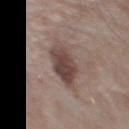The lesion was photographed on a routine skin check and not biopsied; there is no pathology result. From the upper back. A 15 mm crop from a total-body photograph taken for skin-cancer surveillance. Automated tile analysis of the lesion measured a nevus-likeness score of about 85/100 and lesion-presence confidence of about 100/100. A male patient, approximately 55 years of age. The lesion's longest dimension is about 5 mm. Captured under white-light illumination.